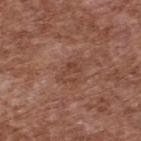Q: Is there a histopathology result?
A: imaged on a skin check; not biopsied
Q: What kind of image is this?
A: ~15 mm crop, total-body skin-cancer survey
Q: Lesion size?
A: about 3 mm
Q: What are the patient's age and sex?
A: male, in their mid- to late 70s
Q: What is the anatomic site?
A: the upper back